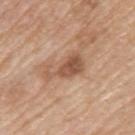follow-up: catalogued during a skin exam; not biopsied | image source: ~15 mm crop, total-body skin-cancer survey | subject: male, aged around 75 | size: ~4.5 mm (longest diameter) | illumination: white-light | anatomic site: the left upper arm | automated lesion analysis: a footprint of about 8.5 mm², an outline eccentricity of about 0.85 (0 = round, 1 = elongated), and two-axis asymmetry of about 0.5; a mean CIELAB color near L≈54 a*≈21 b*≈31, a lesion–skin lightness drop of about 11, and a normalized lesion–skin contrast near 7.5; a color-variation rating of about 4/10 and radial color variation of about 1.5; a classifier nevus-likeness of about 35/100 and a lesion-detection confidence of about 100/100.